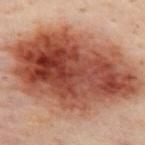image source: 15 mm crop, total-body photography
lighting: cross-polarized
lesion diameter: ~15 mm (longest diameter)
subject: male, roughly 50 years of age
location: the mid back